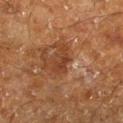Clinical impression: Imaged during a routine full-body skin examination; the lesion was not biopsied and no histopathology is available. Background: On the right lower leg. The patient is a male approximately 60 years of age. Cropped from a total-body skin-imaging series; the visible field is about 15 mm.